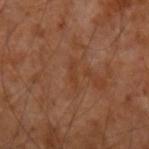biopsy status = imaged on a skin check; not biopsied | site = the left forearm | acquisition = ~15 mm tile from a whole-body skin photo | subject = male, aged 53 to 57.